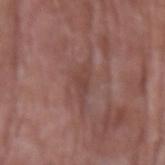Q: Was a biopsy performed?
A: imaged on a skin check; not biopsied
Q: How large is the lesion?
A: ≈3.5 mm
Q: What are the patient's age and sex?
A: male, roughly 65 years of age
Q: How was this image acquired?
A: ~15 mm tile from a whole-body skin photo
Q: Lesion location?
A: the left upper arm